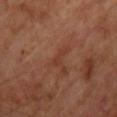{
  "biopsy_status": "not biopsied; imaged during a skin examination",
  "site": "chest",
  "automated_metrics": {
    "area_mm2_approx": 2.5,
    "eccentricity": 0.9,
    "border_irregularity_0_10": 5.5,
    "color_variation_0_10": 0.0,
    "peripheral_color_asymmetry": 0.0
  },
  "lighting": "cross-polarized",
  "lesion_size": {
    "long_diameter_mm_approx": 2.5
  },
  "patient": {
    "sex": "male",
    "age_approx": 65
  },
  "image": {
    "source": "total-body photography crop",
    "field_of_view_mm": 15
  }
}